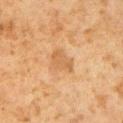<lesion>
  <biopsy_status>not biopsied; imaged during a skin examination</biopsy_status>
  <lesion_size>
    <long_diameter_mm_approx>3.0</long_diameter_mm_approx>
  </lesion_size>
  <automated_metrics>
    <eccentricity>0.6</eccentricity>
    <shape_asymmetry>0.35</shape_asymmetry>
    <cielab_L>51</cielab_L>
    <cielab_a>18</cielab_a>
    <cielab_b>35</cielab_b>
    <vs_skin_contrast_norm>5.5</vs_skin_contrast_norm>
    <border_irregularity_0_10>3.0</border_irregularity_0_10>
    <color_variation_0_10>1.0</color_variation_0_10>
    <nevus_likeness_0_100>0</nevus_likeness_0_100>
    <lesion_detection_confidence_0_100>100</lesion_detection_confidence_0_100>
  </automated_metrics>
  <lighting>cross-polarized</lighting>
  <patient>
    <sex>male</sex>
    <age_approx>60</age_approx>
  </patient>
  <site>left upper arm</site>
  <image>
    <source>total-body photography crop</source>
    <field_of_view_mm>15</field_of_view_mm>
  </image>
</lesion>